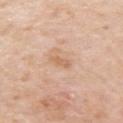Captured during whole-body skin photography for melanoma surveillance; the lesion was not biopsied.
This image is a 15 mm lesion crop taken from a total-body photograph.
Longest diameter approximately 2.5 mm.
A male subject, roughly 75 years of age.
The lesion is located on the left upper arm.
The total-body-photography lesion software estimated a mean CIELAB color near L≈64 a*≈20 b*≈34 and a normalized border contrast of about 6. And it measured border irregularity of about 4 on a 0–10 scale, a within-lesion color-variation index near 0/10, and peripheral color asymmetry of about 0. It also reported a classifier nevus-likeness of about 0/100 and a lesion-detection confidence of about 100/100.